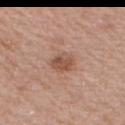This lesion was catalogued during total-body skin photography and was not selected for biopsy. The lesion is on the back. The tile uses white-light illumination. A male subject, in their mid-60s. The lesion's longest dimension is about 3 mm. A close-up tile cropped from a whole-body skin photograph, about 15 mm across. The total-body-photography lesion software estimated a lesion area of about 5.5 mm², a shape eccentricity near 0.6, and a symmetry-axis asymmetry near 0.1. And it measured a mean CIELAB color near L≈52 a*≈21 b*≈29 and a lesion–skin lightness drop of about 10. And it measured a lesion-detection confidence of about 100/100.